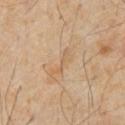acquisition = ~15 mm crop, total-body skin-cancer survey
automated lesion analysis = border irregularity of about 7.5 on a 0–10 scale, a within-lesion color-variation index near 0/10, and radial color variation of about 0; a lesion-detection confidence of about 100/100
illumination = cross-polarized illumination
subject = male, aged 58–62
location = the abdomen
lesion size = ≈3.5 mm